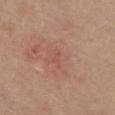A female patient, in their mid- to late 40s. A 15 mm crop from a total-body photograph taken for skin-cancer surveillance. From the chest. Histopathologically confirmed as a superficial basal cell carcinoma (malignant).